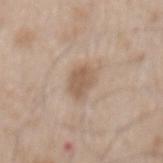Q: Was a biopsy performed?
A: no biopsy performed (imaged during a skin exam)
Q: How was the tile lit?
A: white-light illumination
Q: Where on the body is the lesion?
A: the mid back
Q: What kind of image is this?
A: 15 mm crop, total-body photography
Q: How large is the lesion?
A: ≈3.5 mm
Q: What did automated image analysis measure?
A: a mean CIELAB color near L≈57 a*≈15 b*≈29, a lesion–skin lightness drop of about 10, and a lesion-to-skin contrast of about 7 (normalized; higher = more distinct); border irregularity of about 2.5 on a 0–10 scale, a within-lesion color-variation index near 2/10, and radial color variation of about 0.5
Q: What are the patient's age and sex?
A: male, roughly 50 years of age